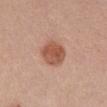Captured during whole-body skin photography for melanoma surveillance; the lesion was not biopsied. The patient is a female aged around 35. About 4 mm across. From the chest. This image is a 15 mm lesion crop taken from a total-body photograph.